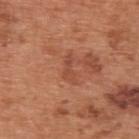biopsy status: total-body-photography surveillance lesion; no biopsy
subject: male, approximately 65 years of age
illumination: white-light
site: the upper back
image-analysis metrics: an area of roughly 3 mm² and a symmetry-axis asymmetry near 0.5; a classifier nevus-likeness of about 0/100 and a lesion-detection confidence of about 100/100
imaging modality: total-body-photography crop, ~15 mm field of view